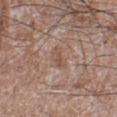Recorded during total-body skin imaging; not selected for excision or biopsy. Measured at roughly 2.5 mm in maximum diameter. The lesion is located on the left lower leg. A close-up tile cropped from a whole-body skin photograph, about 15 mm across. A male subject, aged approximately 60.